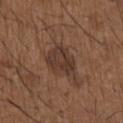workup = catalogued during a skin exam; not biopsied | body site = the right upper arm | lesion diameter = ≈4.5 mm | patient = male, aged around 50 | image = total-body-photography crop, ~15 mm field of view | automated metrics = a footprint of about 11 mm², an eccentricity of roughly 0.65, and two-axis asymmetry of about 0.2; a lesion-detection confidence of about 100/100.